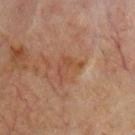Imaged with cross-polarized lighting.
Automated image analysis of the tile measured a footprint of about 4.5 mm², an outline eccentricity of about 0.75 (0 = round, 1 = elongated), and a shape-asymmetry score of about 0.6 (0 = symmetric). It also reported roughly 5 lightness units darker than nearby skin. It also reported a border-irregularity index near 7.5/10 and peripheral color asymmetry of about 0.
From the upper back.
A male patient, aged 63 to 67.
Cropped from a total-body skin-imaging series; the visible field is about 15 mm.